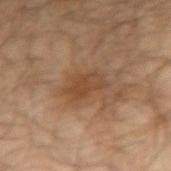Q: Is there a histopathology result?
A: no biopsy performed (imaged during a skin exam)
Q: What is the imaging modality?
A: 15 mm crop, total-body photography
Q: What did automated image analysis measure?
A: an average lesion color of about L≈42 a*≈18 b*≈30 (CIELAB) and a lesion-to-skin contrast of about 6.5 (normalized; higher = more distinct)
Q: Who is the patient?
A: male, about 65 years old
Q: Where on the body is the lesion?
A: the arm
Q: How large is the lesion?
A: about 4 mm